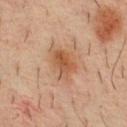Notes:
- biopsy status · total-body-photography surveillance lesion; no biopsy
- body site · the chest
- patient · male, aged around 35
- acquisition · total-body-photography crop, ~15 mm field of view